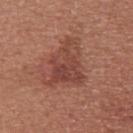Impression: Part of a total-body skin-imaging series; this lesion was reviewed on a skin check and was not flagged for biopsy.